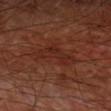Imaged during a routine full-body skin examination; the lesion was not biopsied and no histopathology is available. Located on the arm. The recorded lesion diameter is about 5 mm. This is a cross-polarized tile. This image is a 15 mm lesion crop taken from a total-body photograph. The subject is a male aged approximately 60.